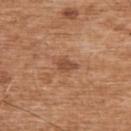Q: Was this lesion biopsied?
A: imaged on a skin check; not biopsied
Q: What is the anatomic site?
A: the upper back
Q: What is the imaging modality?
A: ~15 mm crop, total-body skin-cancer survey
Q: How large is the lesion?
A: about 2.5 mm
Q: What lighting was used for the tile?
A: white-light illumination
Q: What are the patient's age and sex?
A: male, in their mid- to late 70s